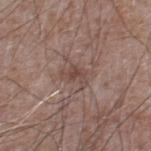Captured during whole-body skin photography for melanoma surveillance; the lesion was not biopsied.